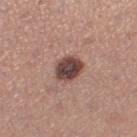Impression: This lesion was catalogued during total-body skin photography and was not selected for biopsy. Acquisition and patient details: A female subject in their 30s. Imaged with white-light lighting. The lesion's longest dimension is about 3.5 mm. This image is a 15 mm lesion crop taken from a total-body photograph. The lesion is on the left thigh.